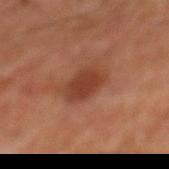Findings:
- notes — total-body-photography surveillance lesion; no biopsy
- patient — male, approximately 60 years of age
- imaging modality — ~15 mm tile from a whole-body skin photo
- site — the chest